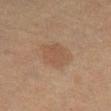Imaged during a routine full-body skin examination; the lesion was not biopsied and no histopathology is available.
Longest diameter approximately 4.5 mm.
From the right lower leg.
A female patient, aged 53 to 57.
A 15 mm close-up tile from a total-body photography series done for melanoma screening.
Automated image analysis of the tile measured an eccentricity of roughly 0.7 and a shape-asymmetry score of about 0.25 (0 = symmetric). And it measured about 5 CIELAB-L* units darker than the surrounding skin and a normalized lesion–skin contrast near 5. The analysis additionally found a border-irregularity index near 2.5/10 and internal color variation of about 2.5 on a 0–10 scale.